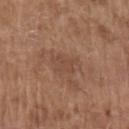The lesion was photographed on a routine skin check and not biopsied; there is no pathology result. Captured under white-light illumination. A region of skin cropped from a whole-body photographic capture, roughly 15 mm wide. An algorithmic analysis of the crop reported a mean CIELAB color near L≈46 a*≈19 b*≈28. The software also gave a peripheral color-asymmetry measure near 0.5. The analysis additionally found an automated nevus-likeness rating near 0 out of 100 and a lesion-detection confidence of about 100/100. The subject is a male aged 78 to 82. On the left upper arm. Approximately 3 mm at its widest.